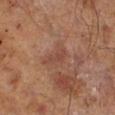<lesion>
<biopsy_status>not biopsied; imaged during a skin examination</biopsy_status>
<automated_metrics>
  <border_irregularity_0_10>4.5</border_irregularity_0_10>
  <color_variation_0_10>0.0</color_variation_0_10>
  <peripheral_color_asymmetry>0.0</peripheral_color_asymmetry>
</automated_metrics>
<site>right lower leg</site>
<patient>
  <sex>male</sex>
  <age_approx>70</age_approx>
</patient>
<lesion_size>
  <long_diameter_mm_approx>3.0</long_diameter_mm_approx>
</lesion_size>
<image>
  <source>total-body photography crop</source>
  <field_of_view_mm>15</field_of_view_mm>
</image>
</lesion>